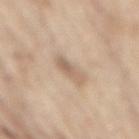biopsy status = catalogued during a skin exam; not biopsied
patient = male, in their 70s
imaging modality = 15 mm crop, total-body photography
body site = the mid back